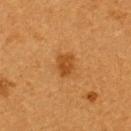Cropped from a total-body skin-imaging series; the visible field is about 15 mm.
From the back.
Measured at roughly 2.5 mm in maximum diameter.
A female patient, in their mid- to late 50s.
Captured under cross-polarized illumination.